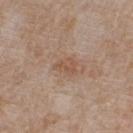follow-up = no biopsy performed (imaged during a skin exam) | patient = male, about 65 years old | site = the chest | automated metrics = a shape eccentricity near 0.75 and two-axis asymmetry of about 0.35; a nevus-likeness score of about 0/100 and a detector confidence of about 100 out of 100 that the crop contains a lesion | diameter = ≈2.5 mm | lighting = white-light illumination | acquisition = 15 mm crop, total-body photography.